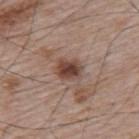Q: How was the tile lit?
A: white-light illumination
Q: How large is the lesion?
A: ~3 mm (longest diameter)
Q: What is the anatomic site?
A: the upper back
Q: Patient demographics?
A: male, about 65 years old
Q: What kind of image is this?
A: total-body-photography crop, ~15 mm field of view